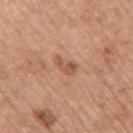Q: Was a biopsy performed?
A: imaged on a skin check; not biopsied
Q: Who is the patient?
A: male, aged approximately 65
Q: Illumination type?
A: white-light
Q: What kind of image is this?
A: ~15 mm tile from a whole-body skin photo
Q: Lesion location?
A: the arm